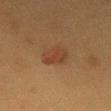The lesion was tiled from a total-body skin photograph and was not biopsied. The lesion is on the chest. An algorithmic analysis of the crop reported two-axis asymmetry of about 0.25. The software also gave about 6 CIELAB-L* units darker than the surrounding skin and a normalized lesion–skin contrast near 6. And it measured internal color variation of about 3 on a 0–10 scale and a peripheral color-asymmetry measure near 1. The analysis additionally found an automated nevus-likeness rating near 75 out of 100 and lesion-presence confidence of about 100/100. A female patient aged around 50. A roughly 15 mm field-of-view crop from a total-body skin photograph. This is a cross-polarized tile.